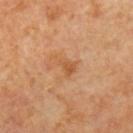biopsy status: imaged on a skin check; not biopsied | body site: the right upper arm | subject: female, in their 50s | imaging modality: ~15 mm crop, total-body skin-cancer survey | automated lesion analysis: a lesion color around L≈54 a*≈22 b*≈38 in CIELAB, about 7 CIELAB-L* units darker than the surrounding skin, and a normalized border contrast of about 6; border irregularity of about 5 on a 0–10 scale, a color-variation rating of about 2/10, and a peripheral color-asymmetry measure near 0.5.